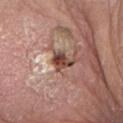image:
  source: total-body photography crop
  field_of_view_mm: 15
automated_metrics:
  border_irregularity_0_10: 3.5
  color_variation_0_10: 4.0
  peripheral_color_asymmetry: 1.0
  nevus_likeness_0_100: 5
  lesion_detection_confidence_0_100: 100
lighting: white-light
patient:
  sex: female
  age_approx: 75
site: left forearm